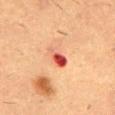Case summary:
* workup · total-body-photography surveillance lesion; no biopsy
* imaging modality · 15 mm crop, total-body photography
* lesion size · ≈3 mm
* tile lighting · cross-polarized illumination
* anatomic site · the mid back
* subject · male, aged around 55